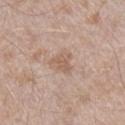An algorithmic analysis of the crop reported a border-irregularity rating of about 5.5/10 and peripheral color asymmetry of about 0.5. The analysis additionally found an automated nevus-likeness rating near 0 out of 100. Captured under white-light illumination. The patient is a male aged 43–47. About 3 mm across. A region of skin cropped from a whole-body photographic capture, roughly 15 mm wide. Located on the right lower leg.